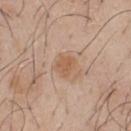{
  "biopsy_status": "not biopsied; imaged during a skin examination",
  "lesion_size": {
    "long_diameter_mm_approx": 2.5
  },
  "patient": {
    "sex": "male",
    "age_approx": 45
  },
  "automated_metrics": {
    "border_irregularity_0_10": 2.5,
    "peripheral_color_asymmetry": 0.5,
    "nevus_likeness_0_100": 35,
    "lesion_detection_confidence_0_100": 100
  },
  "site": "front of the torso",
  "lighting": "white-light",
  "image": {
    "source": "total-body photography crop",
    "field_of_view_mm": 15
  }
}